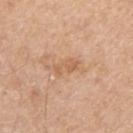imaging modality: total-body-photography crop, ~15 mm field of view; location: the right upper arm; patient: male, aged approximately 70.